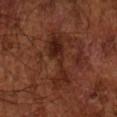Recorded during total-body skin imaging; not selected for excision or biopsy. A male subject in their mid-60s. This image is a 15 mm lesion crop taken from a total-body photograph. Located on the left arm. Automated image analysis of the tile measured a lesion–skin lightness drop of about 7. And it measured a border-irregularity index near 8.5/10, a within-lesion color-variation index near 4/10, and radial color variation of about 1. The analysis additionally found a classifier nevus-likeness of about 0/100 and a lesion-detection confidence of about 100/100.